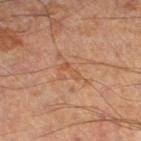{"biopsy_status": "not biopsied; imaged during a skin examination", "site": "left lower leg", "patient": {"sex": "male", "age_approx": 65}, "image": {"source": "total-body photography crop", "field_of_view_mm": 15}}